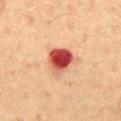Recorded during total-body skin imaging; not selected for excision or biopsy.
Automated tile analysis of the lesion measured a lesion area of about 9 mm², an outline eccentricity of about 0.35 (0 = round, 1 = elongated), and a shape-asymmetry score of about 0.2 (0 = symmetric). It also reported a mean CIELAB color near L≈46 a*≈33 b*≈30, a lesion–skin lightness drop of about 19, and a normalized border contrast of about 13. It also reported a color-variation rating of about 8/10 and radial color variation of about 2. The software also gave a classifier nevus-likeness of about 0/100 and a detector confidence of about 100 out of 100 that the crop contains a lesion.
The lesion is on the abdomen.
Approximately 3.5 mm at its widest.
A male subject, in their 60s.
A close-up tile cropped from a whole-body skin photograph, about 15 mm across.
Imaged with cross-polarized lighting.